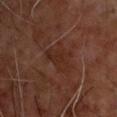follow-up: total-body-photography surveillance lesion; no biopsy | subject: male, approximately 55 years of age | acquisition: total-body-photography crop, ~15 mm field of view | diameter: ≈4 mm | location: the back | TBP lesion metrics: a mean CIELAB color near L≈25 a*≈19 b*≈24, about 5 CIELAB-L* units darker than the surrounding skin, and a normalized lesion–skin contrast near 6; border irregularity of about 5.5 on a 0–10 scale, a color-variation rating of about 2/10, and radial color variation of about 0.5; a classifier nevus-likeness of about 0/100 and a lesion-detection confidence of about 100/100.